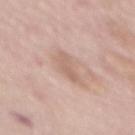Q: Is there a histopathology result?
A: no biopsy performed (imaged during a skin exam)
Q: Automated lesion metrics?
A: a lesion area of about 5 mm², a shape eccentricity near 0.95, and a symmetry-axis asymmetry near 0.3; about 8 CIELAB-L* units darker than the surrounding skin and a normalized border contrast of about 5.5; an automated nevus-likeness rating near 0 out of 100 and a detector confidence of about 100 out of 100 that the crop contains a lesion
Q: What kind of image is this?
A: ~15 mm crop, total-body skin-cancer survey
Q: Who is the patient?
A: female, roughly 65 years of age
Q: Lesion location?
A: the mid back
Q: Lesion size?
A: ~4 mm (longest diameter)
Q: Illumination type?
A: white-light illumination